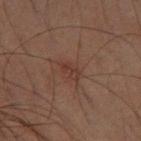{"biopsy_status": "not biopsied; imaged during a skin examination", "image": {"source": "total-body photography crop", "field_of_view_mm": 15}, "lesion_size": {"long_diameter_mm_approx": 3.0}, "patient": {"sex": "male", "age_approx": 60}, "site": "right thigh"}